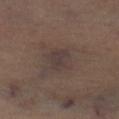Case summary:
* lesion size: ~3.5 mm (longest diameter)
* tile lighting: cross-polarized
* patient: female, about 70 years old
* image: ~15 mm tile from a whole-body skin photo
* body site: the leg
* TBP lesion metrics: a footprint of about 8.5 mm², a shape eccentricity near 0.5, and two-axis asymmetry of about 0.2; a lesion color around L≈37 a*≈12 b*≈17 in CIELAB and a normalized border contrast of about 6; border irregularity of about 2.5 on a 0–10 scale and peripheral color asymmetry of about 1; a nevus-likeness score of about 0/100